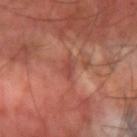size — ~2.5 mm (longest diameter) | image — ~15 mm crop, total-body skin-cancer survey | illumination — cross-polarized illumination | location — the arm | patient — male, aged approximately 60 | automated lesion analysis — a lesion color around L≈45 a*≈27 b*≈27 in CIELAB and a lesion-to-skin contrast of about 6 (normalized; higher = more distinct); border irregularity of about 3.5 on a 0–10 scale, a color-variation rating of about 1.5/10, and peripheral color asymmetry of about 0.5; a detector confidence of about 70 out of 100 that the crop contains a lesion.